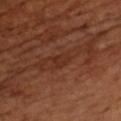notes: catalogued during a skin exam; not biopsied | patient: male, about 65 years old | image source: ~15 mm tile from a whole-body skin photo | size: ~2.5 mm (longest diameter).